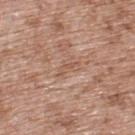workup: total-body-photography surveillance lesion; no biopsy
lesion size: ~3 mm (longest diameter)
acquisition: ~15 mm crop, total-body skin-cancer survey
TBP lesion metrics: a mean CIELAB color near L≈55 a*≈20 b*≈29 and about 7 CIELAB-L* units darker than the surrounding skin; a border-irregularity index near 6.5/10 and a color-variation rating of about 0/10
patient: male, aged 53 to 57
location: the upper back